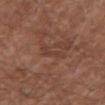Findings:
* follow-up · catalogued during a skin exam; not biopsied
* body site · the right upper arm
* imaging modality · total-body-photography crop, ~15 mm field of view
* patient · male, aged 83 or older
* lighting · white-light illumination